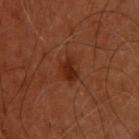<lesion>
<biopsy_status>not biopsied; imaged during a skin examination</biopsy_status>
<automated_metrics>
  <area_mm2_approx>4.0</area_mm2_approx>
  <shape_asymmetry>0.25</shape_asymmetry>
  <vs_skin_contrast_norm>8.0</vs_skin_contrast_norm>
  <nevus_likeness_0_100>80</nevus_likeness_0_100>
  <lesion_detection_confidence_0_100>100</lesion_detection_confidence_0_100>
</automated_metrics>
<site>upper back</site>
<lighting>cross-polarized</lighting>
<lesion_size>
  <long_diameter_mm_approx>2.5</long_diameter_mm_approx>
</lesion_size>
<patient>
  <sex>male</sex>
  <age_approx>55</age_approx>
</patient>
<image>
  <source>total-body photography crop</source>
  <field_of_view_mm>15</field_of_view_mm>
</image>
</lesion>